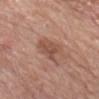Recorded during total-body skin imaging; not selected for excision or biopsy.
This is a white-light tile.
From the head or neck.
A male subject aged approximately 80.
Automated tile analysis of the lesion measured a lesion color around L≈51 a*≈22 b*≈28 in CIELAB, about 9 CIELAB-L* units darker than the surrounding skin, and a normalized border contrast of about 6.5. And it measured border irregularity of about 3 on a 0–10 scale, a within-lesion color-variation index near 3/10, and a peripheral color-asymmetry measure near 1.
A region of skin cropped from a whole-body photographic capture, roughly 15 mm wide.